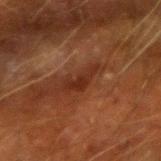<lesion>
  <biopsy_status>not biopsied; imaged during a skin examination</biopsy_status>
  <lesion_size>
    <long_diameter_mm_approx>4.0</long_diameter_mm_approx>
  </lesion_size>
  <site>left forearm</site>
  <patient>
    <sex>male</sex>
    <age_approx>65</age_approx>
  </patient>
  <image>
    <source>total-body photography crop</source>
    <field_of_view_mm>15</field_of_view_mm>
  </image>
  <lighting>cross-polarized</lighting>
</lesion>